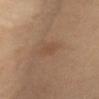This lesion was catalogued during total-body skin photography and was not selected for biopsy. This is a cross-polarized tile. The patient is a female aged approximately 55. About 3 mm across. A roughly 15 mm field-of-view crop from a total-body skin photograph. The lesion is located on the right upper arm. The lesion-visualizer software estimated a footprint of about 4 mm² and a shape-asymmetry score of about 0.25 (0 = symmetric). It also reported a lesion–skin lightness drop of about 6.